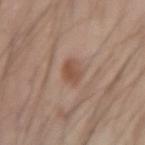This lesion was catalogued during total-body skin photography and was not selected for biopsy.
The subject is a male aged 43 to 47.
This image is a 15 mm lesion crop taken from a total-body photograph.
The lesion is on the left forearm.
The lesion's longest dimension is about 2.5 mm.
Imaged with white-light lighting.
The total-body-photography lesion software estimated a mean CIELAB color near L≈51 a*≈20 b*≈28 and a normalized border contrast of about 7.